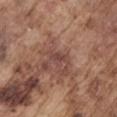biopsy status — imaged on a skin check; not biopsied | image — 15 mm crop, total-body photography | subject — male, approximately 75 years of age | lesion diameter — about 2.5 mm | anatomic site — the right upper arm.